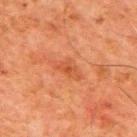{
  "image": {
    "source": "total-body photography crop",
    "field_of_view_mm": 15
  },
  "patient": {
    "sex": "male",
    "age_approx": 80
  },
  "site": "mid back"
}